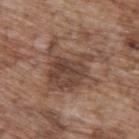Recorded during total-body skin imaging; not selected for excision or biopsy. The lesion is on the upper back. The total-body-photography lesion software estimated a footprint of about 26 mm², an outline eccentricity of about 0.5 (0 = round, 1 = elongated), and two-axis asymmetry of about 0.4. And it measured a nevus-likeness score of about 15/100 and lesion-presence confidence of about 100/100. A male patient about 70 years old. A 15 mm crop from a total-body photograph taken for skin-cancer surveillance. About 7.5 mm across.